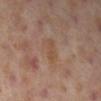Case summary:
- notes — total-body-photography surveillance lesion; no biopsy
- diameter — about 3.5 mm
- acquisition — ~15 mm tile from a whole-body skin photo
- lighting — cross-polarized
- subject — female, approximately 40 years of age
- site — the leg